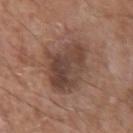Captured during whole-body skin photography for melanoma surveillance; the lesion was not biopsied.
A close-up tile cropped from a whole-body skin photograph, about 15 mm across.
On the left upper arm.
Longest diameter approximately 6.5 mm.
The patient is a male aged 78–82.
The lesion-visualizer software estimated an average lesion color of about L≈43 a*≈18 b*≈24 (CIELAB) and a normalized lesion–skin contrast near 8. It also reported a border-irregularity index near 3/10, a within-lesion color-variation index near 5/10, and radial color variation of about 1.5.
Captured under white-light illumination.